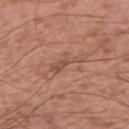biopsy status: total-body-photography surveillance lesion; no biopsy
subject: male, about 40 years old
body site: the right upper arm
imaging modality: 15 mm crop, total-body photography